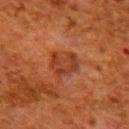notes = no biopsy performed (imaged during a skin exam)
automated lesion analysis = a peripheral color-asymmetry measure near 1; an automated nevus-likeness rating near 25 out of 100 and a lesion-detection confidence of about 100/100
patient = female, in their 50s
image = 15 mm crop, total-body photography
site = the upper back
illumination = cross-polarized illumination